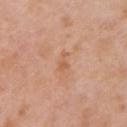Case summary:
- notes — catalogued during a skin exam; not biopsied
- body site — the arm
- diameter — ~2.5 mm (longest diameter)
- patient — female, approximately 40 years of age
- image — 15 mm crop, total-body photography